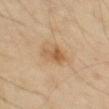Q: Is there a histopathology result?
A: imaged on a skin check; not biopsied
Q: Lesion location?
A: the left thigh
Q: What lighting was used for the tile?
A: cross-polarized
Q: What are the patient's age and sex?
A: male, aged 68–72
Q: What is the imaging modality?
A: ~15 mm crop, total-body skin-cancer survey
Q: Lesion size?
A: ~3.5 mm (longest diameter)